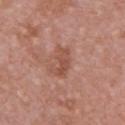Captured during whole-body skin photography for melanoma surveillance; the lesion was not biopsied.
Captured under white-light illumination.
About 3.5 mm across.
The subject is a female in their 40s.
A 15 mm crop from a total-body photograph taken for skin-cancer surveillance.
From the chest.
Automated tile analysis of the lesion measured an area of roughly 5.5 mm², an outline eccentricity of about 0.85 (0 = round, 1 = elongated), and a symmetry-axis asymmetry near 0.35. And it measured a border-irregularity index near 4.5/10, a within-lesion color-variation index near 3.5/10, and peripheral color asymmetry of about 1. The analysis additionally found a nevus-likeness score of about 0/100 and a detector confidence of about 100 out of 100 that the crop contains a lesion.